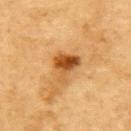{
  "biopsy_status": "not biopsied; imaged during a skin examination",
  "site": "upper back",
  "patient": {
    "sex": "male",
    "age_approx": "85+"
  },
  "image": {
    "source": "total-body photography crop",
    "field_of_view_mm": 15
  }
}